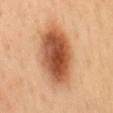No biopsy was performed on this lesion — it was imaged during a full skin examination and was not determined to be concerning. The lesion-visualizer software estimated a border-irregularity index near 1.5/10, internal color variation of about 8.5 on a 0–10 scale, and radial color variation of about 2.5. It also reported a nevus-likeness score of about 100/100 and lesion-presence confidence of about 100/100. A lesion tile, about 15 mm wide, cut from a 3D total-body photograph. From the mid back. A female subject aged around 50. This is a cross-polarized tile.